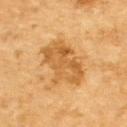The lesion was tiled from a total-body skin photograph and was not biopsied. Approximately 6.5 mm at its widest. A roughly 15 mm field-of-view crop from a total-body skin photograph. This is a cross-polarized tile. Located on the back. The subject is a male aged approximately 85 or older. Automated tile analysis of the lesion measured an area of roughly 17 mm², an eccentricity of roughly 0.8, and a symmetry-axis asymmetry near 0.25. It also reported a lesion–skin lightness drop of about 9. The analysis additionally found a border-irregularity rating of about 4.5/10 and a peripheral color-asymmetry measure near 1.5.